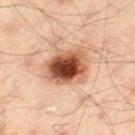Q: Was this lesion biopsied?
A: no biopsy performed (imaged during a skin exam)
Q: Illumination type?
A: cross-polarized illumination
Q: How large is the lesion?
A: about 4.5 mm
Q: Patient demographics?
A: male, about 45 years old
Q: What is the anatomic site?
A: the left thigh
Q: What kind of image is this?
A: total-body-photography crop, ~15 mm field of view